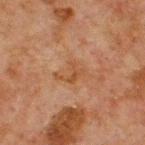biopsy status: no biopsy performed (imaged during a skin exam) | illumination: cross-polarized illumination | patient: male, in their mid- to late 60s | acquisition: 15 mm crop, total-body photography | size: ~4 mm (longest diameter) | TBP lesion metrics: a footprint of about 6.5 mm², an outline eccentricity of about 0.8 (0 = round, 1 = elongated), and a symmetry-axis asymmetry near 0.4; a nevus-likeness score of about 0/100 and a lesion-detection confidence of about 100/100 | body site: the chest.